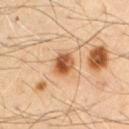{"biopsy_status": "not biopsied; imaged during a skin examination", "image": {"source": "total-body photography crop", "field_of_view_mm": 15}, "site": "chest", "patient": {"sex": "male", "age_approx": 55}, "lesion_size": {"long_diameter_mm_approx": 4.0}, "lighting": "cross-polarized", "automated_metrics": {"cielab_L": 59, "cielab_a": 21, "cielab_b": 37, "vs_skin_darker_L": 11.0, "vs_skin_contrast_norm": 7.5, "border_irregularity_0_10": 3.5, "color_variation_0_10": 10.0, "peripheral_color_asymmetry": 3.0, "nevus_likeness_0_100": 100}}